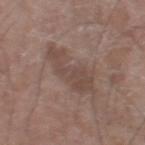Clinical impression: Captured during whole-body skin photography for melanoma surveillance; the lesion was not biopsied. Context: Longest diameter approximately 7 mm. Located on the left lower leg. A male patient, aged 58 to 62. An algorithmic analysis of the crop reported a footprint of about 15 mm², an outline eccentricity of about 0.9 (0 = round, 1 = elongated), and a symmetry-axis asymmetry near 0.35. It also reported radial color variation of about 1. The software also gave a classifier nevus-likeness of about 0/100 and a lesion-detection confidence of about 100/100. A 15 mm crop from a total-body photograph taken for skin-cancer surveillance.